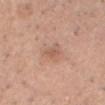biopsy status: no biopsy performed (imaged during a skin exam); acquisition: ~15 mm tile from a whole-body skin photo; lesion diameter: ≈3 mm; location: the head or neck; lighting: white-light illumination; subject: male, approximately 35 years of age.